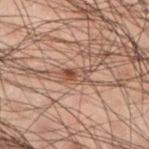Imaged during a routine full-body skin examination; the lesion was not biopsied and no histopathology is available.
An algorithmic analysis of the crop reported an area of roughly 3.5 mm², an outline eccentricity of about 0.9 (0 = round, 1 = elongated), and a shape-asymmetry score of about 0.3 (0 = symmetric). The software also gave an average lesion color of about L≈37 a*≈16 b*≈23 (CIELAB) and a lesion-to-skin contrast of about 7 (normalized; higher = more distinct). It also reported border irregularity of about 3 on a 0–10 scale, a color-variation rating of about 8.5/10, and peripheral color asymmetry of about 3. The analysis additionally found a classifier nevus-likeness of about 95/100 and a lesion-detection confidence of about 100/100.
The lesion is located on the left lower leg.
A male subject aged around 50.
Captured under cross-polarized illumination.
A close-up tile cropped from a whole-body skin photograph, about 15 mm across.
The recorded lesion diameter is about 3 mm.